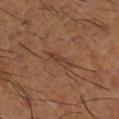Case summary:
* follow-up — total-body-photography surveillance lesion; no biopsy
* acquisition — total-body-photography crop, ~15 mm field of view
* tile lighting — cross-polarized illumination
* patient — male, roughly 65 years of age
* lesion size — about 3.5 mm
* anatomic site — the right lower leg
* TBP lesion metrics — a nevus-likeness score of about 0/100 and lesion-presence confidence of about 75/100